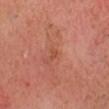Notes:
* biopsy status · total-body-photography surveillance lesion; no biopsy
* acquisition · ~15 mm crop, total-body skin-cancer survey
* tile lighting · cross-polarized illumination
* location · the head or neck
* diameter · ~1.5 mm (longest diameter)
* TBP lesion metrics · a footprint of about 1 mm², an eccentricity of roughly 0.75, and a shape-asymmetry score of about 0.45 (0 = symmetric); a mean CIELAB color near L≈48 a*≈28 b*≈34, about 6 CIELAB-L* units darker than the surrounding skin, and a normalized lesion–skin contrast near 5.5; a border-irregularity rating of about 3/10 and a within-lesion color-variation index near 0/10; a nevus-likeness score of about 0/100
* subject · about 55 years old